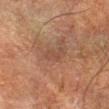Context: Longest diameter approximately 3 mm. A roughly 15 mm field-of-view crop from a total-body skin photograph. The lesion is located on the left forearm. The lesion-visualizer software estimated an eccentricity of roughly 0.9 and a symmetry-axis asymmetry near 0.55. The analysis additionally found a nevus-likeness score of about 0/100 and a detector confidence of about 100 out of 100 that the crop contains a lesion. A male subject, aged 48 to 52.